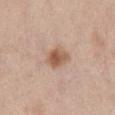Imaged during a routine full-body skin examination; the lesion was not biopsied and no histopathology is available. Cropped from a total-body skin-imaging series; the visible field is about 15 mm. From the back. Imaged with white-light lighting. The patient is a female approximately 40 years of age. The lesion's longest dimension is about 3.5 mm. The total-body-photography lesion software estimated an area of roughly 7 mm², an eccentricity of roughly 0.6, and two-axis asymmetry of about 0.2. And it measured an average lesion color of about L≈57 a*≈19 b*≈30 (CIELAB) and about 11 CIELAB-L* units darker than the surrounding skin. The analysis additionally found a classifier nevus-likeness of about 90/100 and lesion-presence confidence of about 100/100.